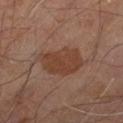follow-up: no biopsy performed (imaged during a skin exam) | TBP lesion metrics: an area of roughly 14 mm², a shape eccentricity near 0.75, and a symmetry-axis asymmetry near 0.25; an average lesion color of about L≈37 a*≈19 b*≈26 (CIELAB), roughly 7 lightness units darker than nearby skin, and a lesion-to-skin contrast of about 7.5 (normalized; higher = more distinct); a within-lesion color-variation index near 2/10 | imaging modality: 15 mm crop, total-body photography | tile lighting: cross-polarized illumination | location: the leg | diameter: ~5.5 mm (longest diameter) | subject: male, aged approximately 55.